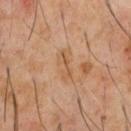Q: Was a biopsy performed?
A: catalogued during a skin exam; not biopsied
Q: Lesion location?
A: the chest
Q: Who is the patient?
A: male, about 60 years old
Q: Automated lesion metrics?
A: a lesion area of about 4.5 mm² and an eccentricity of roughly 0.95; a within-lesion color-variation index near 2.5/10 and radial color variation of about 0.5
Q: What lighting was used for the tile?
A: cross-polarized
Q: How large is the lesion?
A: ≈3.5 mm
Q: What is the imaging modality?
A: 15 mm crop, total-body photography